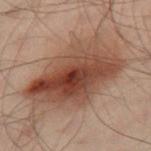workup = no biopsy performed (imaged during a skin exam) | acquisition = ~15 mm crop, total-body skin-cancer survey | subject = male, in their 50s | body site = the leg.